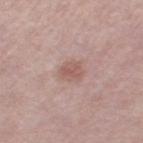The lesion was photographed on a routine skin check and not biopsied; there is no pathology result. Imaged with white-light lighting. On the left thigh. A roughly 15 mm field-of-view crop from a total-body skin photograph. The patient is a female approximately 50 years of age.